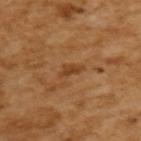Findings:
– notes · total-body-photography surveillance lesion; no biopsy
– lesion size · about 3 mm
– lighting · cross-polarized illumination
– imaging modality · total-body-photography crop, ~15 mm field of view
– subject · male, aged around 65